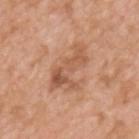biopsy_status: not biopsied; imaged during a skin examination
image:
  source: total-body photography crop
  field_of_view_mm: 15
site: upper back
patient:
  sex: male
  age_approx: 50